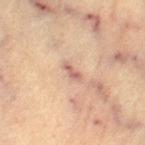This lesion was catalogued during total-body skin photography and was not selected for biopsy.
A lesion tile, about 15 mm wide, cut from a 3D total-body photograph.
A female subject roughly 65 years of age.
On the left thigh.